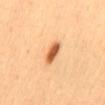<tbp_lesion>
<lighting>cross-polarized</lighting>
<image>
  <source>total-body photography crop</source>
  <field_of_view_mm>15</field_of_view_mm>
</image>
<automated_metrics>
  <border_irregularity_0_10>1.5</border_irregularity_0_10>
  <color_variation_0_10>4.0</color_variation_0_10>
  <peripheral_color_asymmetry>1.5</peripheral_color_asymmetry>
  <nevus_likeness_0_100>100</nevus_likeness_0_100>
  <lesion_detection_confidence_0_100>100</lesion_detection_confidence_0_100>
</automated_metrics>
<lesion_size>
  <long_diameter_mm_approx>3.5</long_diameter_mm_approx>
</lesion_size>
<site>mid back</site>
<patient>
  <sex>female</sex>
  <age_approx>45</age_approx>
</patient>
</tbp_lesion>